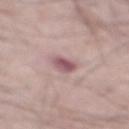{"patient": {"sex": "male", "age_approx": 65}, "lighting": "white-light", "image": {"source": "total-body photography crop", "field_of_view_mm": 15}, "site": "mid back", "lesion_size": {"long_diameter_mm_approx": 2.5}}